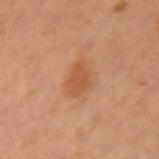Findings:
– lesion size · ~4 mm (longest diameter)
– site · the left upper arm
– image source · total-body-photography crop, ~15 mm field of view
– illumination · cross-polarized illumination
– subject · male, in their 60s